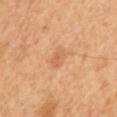Background: The subject is a male aged 58 to 62. About 3 mm across. Located on the chest. This is a cross-polarized tile. A lesion tile, about 15 mm wide, cut from a 3D total-body photograph.